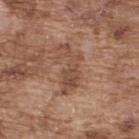Part of a total-body skin-imaging series; this lesion was reviewed on a skin check and was not flagged for biopsy.
Located on the upper back.
A roughly 15 mm field-of-view crop from a total-body skin photograph.
A male patient, in their mid- to late 70s.